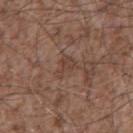Notes:
* notes · total-body-photography surveillance lesion; no biopsy
* lesion size · ~3 mm (longest diameter)
* anatomic site · the mid back
* image · ~15 mm tile from a whole-body skin photo
* subject · male, aged 53 to 57
* automated lesion analysis · an average lesion color of about L≈41 a*≈18 b*≈25 (CIELAB) and a normalized lesion–skin contrast near 5.5; a border-irregularity rating of about 5/10, a color-variation rating of about 1.5/10, and a peripheral color-asymmetry measure near 0.5; a detector confidence of about 60 out of 100 that the crop contains a lesion